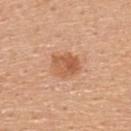Clinical impression: Captured during whole-body skin photography for melanoma surveillance; the lesion was not biopsied. Image and clinical context: A female subject, about 45 years old. Located on the upper back. An algorithmic analysis of the crop reported roughly 10 lightness units darker than nearby skin and a lesion-to-skin contrast of about 7.5 (normalized; higher = more distinct). Captured under white-light illumination. The recorded lesion diameter is about 3.5 mm. A lesion tile, about 15 mm wide, cut from a 3D total-body photograph.